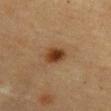  biopsy_status: not biopsied; imaged during a skin examination
  automated_metrics:
    border_irregularity_0_10: 2.0
    color_variation_0_10: 4.0
    peripheral_color_asymmetry: 1.0
    nevus_likeness_0_100: 100
    lesion_detection_confidence_0_100: 100
  lesion_size:
    long_diameter_mm_approx: 3.0
  image:
    source: total-body photography crop
    field_of_view_mm: 15
  lighting: cross-polarized
  patient:
    sex: female
    age_approx: 55
  site: abdomen